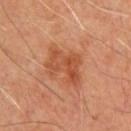workup: imaged on a skin check; not biopsied | subject: male, approximately 55 years of age | size: ≈4.5 mm | image-analysis metrics: an area of roughly 9.5 mm²; a classifier nevus-likeness of about 40/100 and a detector confidence of about 100 out of 100 that the crop contains a lesion | acquisition: ~15 mm crop, total-body skin-cancer survey | site: the chest.